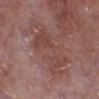biopsy_status: not biopsied; imaged during a skin examination
site: right lower leg
patient:
  sex: male
  age_approx: 75
image:
  source: total-body photography crop
  field_of_view_mm: 15